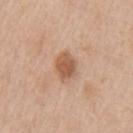Recorded during total-body skin imaging; not selected for excision or biopsy. A region of skin cropped from a whole-body photographic capture, roughly 15 mm wide. The lesion is on the left upper arm. The tile uses white-light illumination. A male subject, aged 58 to 62.